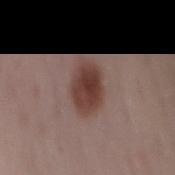Findings:
- workup — catalogued during a skin exam; not biopsied
- image — 15 mm crop, total-body photography
- location — the mid back
- illumination — white-light
- lesion diameter — about 5.5 mm
- patient — female, roughly 50 years of age